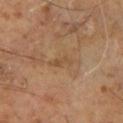notes = imaged on a skin check; not biopsied
lesion diameter = ~2.5 mm (longest diameter)
patient = male, in their 60s
location = the right leg
illumination = cross-polarized illumination
image = ~15 mm tile from a whole-body skin photo